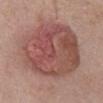Part of a total-body skin-imaging series; this lesion was reviewed on a skin check and was not flagged for biopsy.
Cropped from a whole-body photographic skin survey; the tile spans about 15 mm.
An algorithmic analysis of the crop reported a classifier nevus-likeness of about 100/100.
Imaged with white-light lighting.
A male subject, aged 58–62.
Longest diameter approximately 10.5 mm.
The lesion is located on the abdomen.